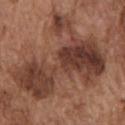Assessment: The lesion was photographed on a routine skin check and not biopsied; there is no pathology result. Acquisition and patient details: The recorded lesion diameter is about 11 mm. Located on the chest. A male patient, approximately 75 years of age. Captured under white-light illumination. Cropped from a total-body skin-imaging series; the visible field is about 15 mm. Automated image analysis of the tile measured an area of roughly 50 mm², a shape eccentricity near 0.8, and two-axis asymmetry of about 0.6. The analysis additionally found an average lesion color of about L≈39 a*≈21 b*≈26 (CIELAB), a lesion–skin lightness drop of about 12, and a normalized border contrast of about 10. It also reported border irregularity of about 9.5 on a 0–10 scale. And it measured an automated nevus-likeness rating near 0 out of 100 and a lesion-detection confidence of about 100/100.